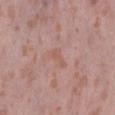Q: Was a biopsy performed?
A: catalogued during a skin exam; not biopsied
Q: What lighting was used for the tile?
A: white-light
Q: What are the patient's age and sex?
A: female, aged 38–42
Q: How was this image acquired?
A: ~15 mm crop, total-body skin-cancer survey
Q: Automated lesion metrics?
A: an outline eccentricity of about 0.9 (0 = round, 1 = elongated); a lesion color around L≈57 a*≈21 b*≈26 in CIELAB and a normalized border contrast of about 5
Q: Lesion location?
A: the abdomen
Q: What is the lesion's diameter?
A: ≈3 mm